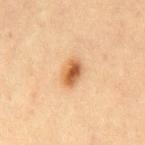Recorded during total-body skin imaging; not selected for excision or biopsy.
This is a cross-polarized tile.
A 15 mm crop from a total-body photograph taken for skin-cancer surveillance.
A male subject, aged 43 to 47.
From the mid back.
The lesion-visualizer software estimated a footprint of about 5.5 mm². The analysis additionally found a lesion color around L≈52 a*≈21 b*≈37 in CIELAB and a lesion–skin lightness drop of about 13. The software also gave a classifier nevus-likeness of about 100/100 and a lesion-detection confidence of about 100/100.